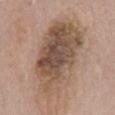Clinical impression: Recorded during total-body skin imaging; not selected for excision or biopsy. Acquisition and patient details: A 15 mm crop from a total-body photograph taken for skin-cancer surveillance. The lesion is located on the back. A male subject, approximately 60 years of age.